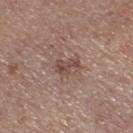Captured during whole-body skin photography for melanoma surveillance; the lesion was not biopsied. A female subject, aged around 65. Cropped from a total-body skin-imaging series; the visible field is about 15 mm. The lesion is on the right thigh.